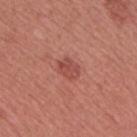Captured during whole-body skin photography for melanoma surveillance; the lesion was not biopsied. A male patient roughly 45 years of age. Located on the chest. A 15 mm close-up extracted from a 3D total-body photography capture. The lesion's longest dimension is about 3 mm. This is a white-light tile.